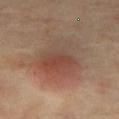The total-body-photography lesion software estimated an area of roughly 21 mm², a shape eccentricity near 0.3, and a symmetry-axis asymmetry near 0.3. And it measured a border-irregularity index near 3/10. It also reported lesion-presence confidence of about 100/100. From the leg. Measured at roughly 5.5 mm in maximum diameter. Captured under cross-polarized illumination. A 15 mm crop from a total-body photograph taken for skin-cancer surveillance. A female subject, about 60 years old.